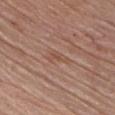The lesion was photographed on a routine skin check and not biopsied; there is no pathology result. A male patient, aged around 75. The tile uses white-light illumination. Measured at roughly 3 mm in maximum diameter. The lesion is on the chest. A region of skin cropped from a whole-body photographic capture, roughly 15 mm wide. An algorithmic analysis of the crop reported a lesion color around L≈51 a*≈20 b*≈28 in CIELAB and roughly 6 lightness units darker than nearby skin. It also reported a nevus-likeness score of about 0/100 and lesion-presence confidence of about 95/100.